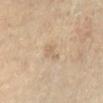Findings:
– notes: total-body-photography surveillance lesion; no biopsy
– imaging modality: 15 mm crop, total-body photography
– lesion size: ≈3 mm
– body site: the left lower leg
– patient: roughly 60 years of age
– illumination: cross-polarized illumination